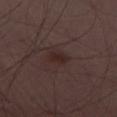{"biopsy_status": "not biopsied; imaged during a skin examination", "lesion_size": {"long_diameter_mm_approx": 3.0}, "automated_metrics": {"area_mm2_approx": 3.0, "eccentricity": 0.85, "shape_asymmetry": 0.25, "vs_skin_darker_L": 6.0, "nevus_likeness_0_100": 20}, "lighting": "white-light", "image": {"source": "total-body photography crop", "field_of_view_mm": 15}, "patient": {"sex": "male", "age_approx": 50}}